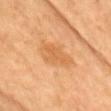Case summary:
* follow-up — total-body-photography surveillance lesion; no biopsy
* body site — the chest
* automated metrics — a lesion area of about 12 mm² and an eccentricity of roughly 0.85; a border-irregularity rating of about 2.5/10 and a within-lesion color-variation index near 2/10; an automated nevus-likeness rating near 5 out of 100 and lesion-presence confidence of about 100/100
* size — ≈5.5 mm
* illumination — cross-polarized
* subject — female, aged approximately 50
* imaging modality — ~15 mm tile from a whole-body skin photo